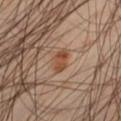workup = no biopsy performed (imaged during a skin exam) | site = the left thigh | subject = male, aged around 45 | image source = total-body-photography crop, ~15 mm field of view | lesion diameter = ≈3 mm.